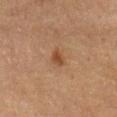Q: Was a biopsy performed?
A: catalogued during a skin exam; not biopsied
Q: Automated lesion metrics?
A: a lesion area of about 3.5 mm², an outline eccentricity of about 0.75 (0 = round, 1 = elongated), and a shape-asymmetry score of about 0.3 (0 = symmetric); a border-irregularity index near 2.5/10, a within-lesion color-variation index near 1.5/10, and peripheral color asymmetry of about 0.5
Q: What is the anatomic site?
A: the mid back
Q: Patient demographics?
A: male, aged 73–77
Q: How was the tile lit?
A: cross-polarized illumination
Q: What is the imaging modality?
A: ~15 mm crop, total-body skin-cancer survey
Q: Lesion size?
A: ~2.5 mm (longest diameter)